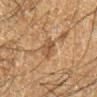| feature | finding |
|---|---|
| notes | imaged on a skin check; not biopsied |
| tile lighting | cross-polarized illumination |
| subject | male, roughly 60 years of age |
| imaging modality | ~15 mm crop, total-body skin-cancer survey |
| anatomic site | the right lower leg |
| lesion size | ~3 mm (longest diameter) |